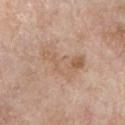Imaged during a routine full-body skin examination; the lesion was not biopsied and no histopathology is available.
From the chest.
The tile uses white-light illumination.
Longest diameter approximately 6 mm.
A 15 mm close-up tile from a total-body photography series done for melanoma screening.
The subject is a male in their 60s.